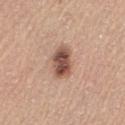biopsy_status: not biopsied; imaged during a skin examination
image:
  source: total-body photography crop
  field_of_view_mm: 15
site: left thigh
automated_metrics:
  cielab_L: 51
  cielab_a: 21
  cielab_b: 27
  vs_skin_darker_L: 16.0
  vs_skin_contrast_norm: 10.5
  color_variation_0_10: 6.0
  peripheral_color_asymmetry: 2.0
patient:
  sex: female
  age_approx: 65